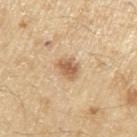  biopsy_status: not biopsied; imaged during a skin examination
  lighting: white-light
  image:
    source: total-body photography crop
    field_of_view_mm: 15
  lesion_size:
    long_diameter_mm_approx: 2.5
  patient:
    sex: male
    age_approx: 70
  site: right upper arm